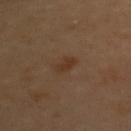Assessment:
Imaged during a routine full-body skin examination; the lesion was not biopsied and no histopathology is available.
Acquisition and patient details:
The total-body-photography lesion software estimated a footprint of about 3.5 mm², an outline eccentricity of about 0.9 (0 = round, 1 = elongated), and a symmetry-axis asymmetry near 0.35. The analysis additionally found a border-irregularity rating of about 3.5/10, a within-lesion color-variation index near 0.5/10, and a peripheral color-asymmetry measure near 0. It also reported a detector confidence of about 100 out of 100 that the crop contains a lesion. A female patient approximately 60 years of age. About 3 mm across. On the chest. A close-up tile cropped from a whole-body skin photograph, about 15 mm across.